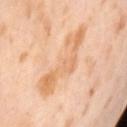<tbp_lesion>
<biopsy_status>not biopsied; imaged during a skin examination</biopsy_status>
<site>back</site>
<patient>
  <sex>female</sex>
  <age_approx>55</age_approx>
</patient>
<lesion_size>
  <long_diameter_mm_approx>8.5</long_diameter_mm_approx>
</lesion_size>
<automated_metrics>
  <lesion_detection_confidence_0_100>100</lesion_detection_confidence_0_100>
</automated_metrics>
<lighting>cross-polarized</lighting>
<image>
  <source>total-body photography crop</source>
  <field_of_view_mm>15</field_of_view_mm>
</image>
</tbp_lesion>